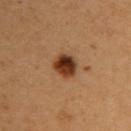Impression:
Recorded during total-body skin imaging; not selected for excision or biopsy.
Image and clinical context:
The lesion is located on the right upper arm. The tile uses cross-polarized illumination. The subject is a female aged 33 to 37. Automated tile analysis of the lesion measured an average lesion color of about L≈36 a*≈22 b*≈33 (CIELAB) and a normalized lesion–skin contrast near 13.5. The analysis additionally found a classifier nevus-likeness of about 100/100 and lesion-presence confidence of about 100/100. About 3 mm across. A close-up tile cropped from a whole-body skin photograph, about 15 mm across.